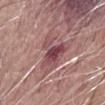{
  "biopsy_status": "not biopsied; imaged during a skin examination",
  "patient": {
    "sex": "male",
    "age_approx": 65
  },
  "lesion_size": {
    "long_diameter_mm_approx": 5.5
  },
  "image": {
    "source": "total-body photography crop",
    "field_of_view_mm": 15
  },
  "site": "arm",
  "automated_metrics": {
    "cielab_L": 49,
    "cielab_a": 24,
    "cielab_b": 17,
    "vs_skin_contrast_norm": 7.5
  },
  "lighting": "white-light"
}